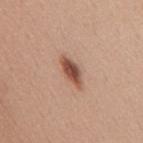* follow-up — total-body-photography surveillance lesion; no biopsy